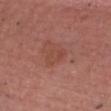{"biopsy_status": "not biopsied; imaged during a skin examination", "lighting": "white-light", "site": "front of the torso", "image": {"source": "total-body photography crop", "field_of_view_mm": 15}, "lesion_size": {"long_diameter_mm_approx": 3.0}, "patient": {"sex": "male", "age_approx": 65}}